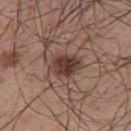follow-up: catalogued during a skin exam; not biopsied
diameter: ≈4 mm
location: the upper back
subject: male, aged approximately 50
image source: 15 mm crop, total-body photography
automated lesion analysis: a footprint of about 8.5 mm², an eccentricity of roughly 0.65, and a symmetry-axis asymmetry near 0.2; a border-irregularity index near 2/10 and a within-lesion color-variation index near 5/10; a classifier nevus-likeness of about 90/100 and a lesion-detection confidence of about 100/100
illumination: white-light